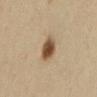Q: Was a biopsy performed?
A: catalogued during a skin exam; not biopsied
Q: What is the anatomic site?
A: the lower back
Q: How was this image acquired?
A: ~15 mm crop, total-body skin-cancer survey
Q: Patient demographics?
A: female, roughly 60 years of age
Q: How large is the lesion?
A: ~3.5 mm (longest diameter)
Q: Automated lesion metrics?
A: an area of roughly 7 mm², a shape eccentricity near 0.4, and a symmetry-axis asymmetry near 0.2; a lesion color around L≈44 a*≈14 b*≈29 in CIELAB and roughly 13 lightness units darker than nearby skin
Q: What lighting was used for the tile?
A: cross-polarized